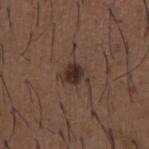Case summary:
– workup · no biopsy performed (imaged during a skin exam)
– automated metrics · a within-lesion color-variation index near 3.5/10 and a peripheral color-asymmetry measure near 1; a nevus-likeness score of about 95/100 and a detector confidence of about 100 out of 100 that the crop contains a lesion
– patient · male, in their 50s
– size · about 3 mm
– image source · ~15 mm crop, total-body skin-cancer survey
– lighting · white-light
– location · the front of the torso